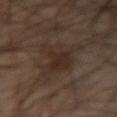Clinical impression: Part of a total-body skin-imaging series; this lesion was reviewed on a skin check and was not flagged for biopsy. Background: The subject is a male roughly 65 years of age. Located on the left forearm. The lesion's longest dimension is about 6.5 mm. A roughly 15 mm field-of-view crop from a total-body skin photograph.